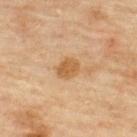Q: Lesion location?
A: the upper back
Q: Who is the patient?
A: male, aged around 45
Q: What is the imaging modality?
A: ~15 mm tile from a whole-body skin photo